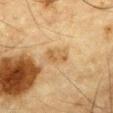follow-up=total-body-photography surveillance lesion; no biopsy
size=about 3 mm
image=15 mm crop, total-body photography
anatomic site=the chest
subject=male, approximately 85 years of age or older